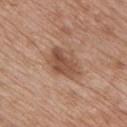Notes:
- workup · catalogued during a skin exam; not biopsied
- site · the chest
- acquisition · ~15 mm tile from a whole-body skin photo
- lesion diameter · ~4.5 mm (longest diameter)
- patient · male, aged around 65
- lighting · white-light illumination